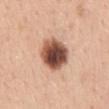<tbp_lesion>
<image>
  <source>total-body photography crop</source>
  <field_of_view_mm>15</field_of_view_mm>
</image>
<patient>
  <sex>female</sex>
  <age_approx>45</age_approx>
</patient>
<lesion_size>
  <long_diameter_mm_approx>5.0</long_diameter_mm_approx>
</lesion_size>
<lighting>white-light</lighting>
<site>mid back</site>
</tbp_lesion>